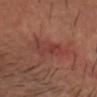| feature | finding |
|---|---|
| biopsy status | imaged on a skin check; not biopsied |
| site | the head or neck |
| imaging modality | ~15 mm crop, total-body skin-cancer survey |
| patient | male, aged around 40 |